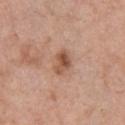Clinical impression:
The lesion was photographed on a routine skin check and not biopsied; there is no pathology result.
Background:
On the chest. Cropped from a whole-body photographic skin survey; the tile spans about 15 mm. The tile uses white-light illumination. A male subject, about 60 years old.